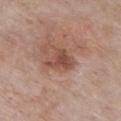notes=catalogued during a skin exam; not biopsied
illumination=white-light illumination
subject=female, approximately 85 years of age or older
automated metrics=a border-irregularity rating of about 4/10 and peripheral color asymmetry of about 1.5
anatomic site=the chest
image=15 mm crop, total-body photography
lesion diameter=about 3.5 mm